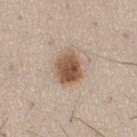The lesion was tiled from a total-body skin photograph and was not biopsied.
A roughly 15 mm field-of-view crop from a total-body skin photograph.
From the right thigh.
The subject is a female approximately 60 years of age.
The tile uses white-light illumination.